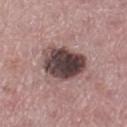Case summary:
- automated metrics: a footprint of about 17 mm² and an outline eccentricity of about 0.55 (0 = round, 1 = elongated)
- patient: female, aged approximately 45
- site: the left thigh
- imaging modality: ~15 mm crop, total-body skin-cancer survey
- lesion diameter: ≈5.5 mm
- tile lighting: white-light illumination